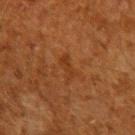Clinical impression: Recorded during total-body skin imaging; not selected for excision or biopsy. Image and clinical context: Located on the right upper arm. A 15 mm close-up extracted from a 3D total-body photography capture. A male patient roughly 60 years of age.